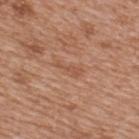Case summary:
- follow-up — catalogued during a skin exam; not biopsied
- patient — male, in their mid- to late 60s
- TBP lesion metrics — a color-variation rating of about 0/10
- imaging modality — ~15 mm crop, total-body skin-cancer survey
- lesion diameter — ~3 mm (longest diameter)
- body site — the upper back